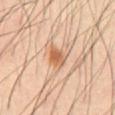* notes — total-body-photography surveillance lesion; no biopsy
* lesion diameter — ≈2.5 mm
* image source — total-body-photography crop, ~15 mm field of view
* anatomic site — the abdomen
* tile lighting — cross-polarized illumination
* patient — male, about 60 years old
* TBP lesion metrics — a lesion area of about 5 mm² and an outline eccentricity of about 0.55 (0 = round, 1 = elongated); a mean CIELAB color near L≈59 a*≈22 b*≈35 and a lesion–skin lightness drop of about 11; a nevus-likeness score of about 85/100 and lesion-presence confidence of about 100/100